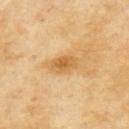Impression:
No biopsy was performed on this lesion — it was imaged during a full skin examination and was not determined to be concerning.
Background:
The subject is a female approximately 60 years of age. The tile uses cross-polarized illumination. The lesion is on the upper back. A 15 mm crop from a total-body photograph taken for skin-cancer surveillance. Automated image analysis of the tile measured a symmetry-axis asymmetry near 0.25. The analysis additionally found a lesion color around L≈58 a*≈17 b*≈42 in CIELAB, about 9 CIELAB-L* units darker than the surrounding skin, and a normalized lesion–skin contrast near 7. It also reported a border-irregularity index near 3/10, a color-variation rating of about 3.5/10, and peripheral color asymmetry of about 1. Longest diameter approximately 4.5 mm.